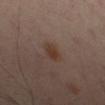Impression:
No biopsy was performed on this lesion — it was imaged during a full skin examination and was not determined to be concerning.
Background:
On the left thigh. Automated tile analysis of the lesion measured a lesion area of about 4 mm² and a shape-asymmetry score of about 0.2 (0 = symmetric). The software also gave a border-irregularity index near 2/10, a within-lesion color-variation index near 2/10, and radial color variation of about 0.5. The subject is a male aged approximately 65. Cropped from a total-body skin-imaging series; the visible field is about 15 mm. Measured at roughly 3 mm in maximum diameter.